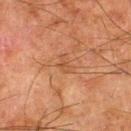Assessment:
Captured during whole-body skin photography for melanoma surveillance; the lesion was not biopsied.
Acquisition and patient details:
Cropped from a whole-body photographic skin survey; the tile spans about 15 mm. The tile uses cross-polarized illumination. Located on the left thigh. The subject is a male in their 80s. The lesion's longest dimension is about 2.5 mm.